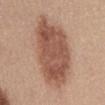The lesion was tiled from a total-body skin photograph and was not biopsied. A female patient, aged 43–47. From the front of the torso. Measured at roughly 9.5 mm in maximum diameter. This image is a 15 mm lesion crop taken from a total-body photograph.